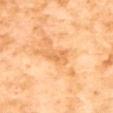Q: Was this lesion biopsied?
A: imaged on a skin check; not biopsied
Q: What lighting was used for the tile?
A: cross-polarized illumination
Q: Patient demographics?
A: female, aged 53–57
Q: What is the imaging modality?
A: 15 mm crop, total-body photography
Q: What did automated image analysis measure?
A: an outline eccentricity of about 0.85 (0 = round, 1 = elongated) and a symmetry-axis asymmetry near 0.5; a classifier nevus-likeness of about 0/100
Q: How large is the lesion?
A: about 3.5 mm
Q: Lesion location?
A: the upper back